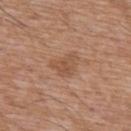The lesion was photographed on a routine skin check and not biopsied; there is no pathology result. A male patient, aged 58 to 62. Approximately 3.5 mm at its widest. The lesion is on the chest. A 15 mm close-up extracted from a 3D total-body photography capture.